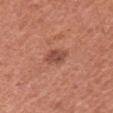  biopsy_status: not biopsied; imaged during a skin examination
  patient:
    sex: female
    age_approx: 50
  image:
    source: total-body photography crop
    field_of_view_mm: 15
  site: left upper arm